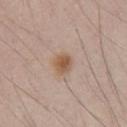{
  "biopsy_status": "not biopsied; imaged during a skin examination",
  "lighting": "white-light",
  "automated_metrics": {
    "lesion_detection_confidence_0_100": 100
  },
  "site": "chest",
  "image": {
    "source": "total-body photography crop",
    "field_of_view_mm": 15
  },
  "patient": {
    "sex": "male",
    "age_approx": 35
  }
}